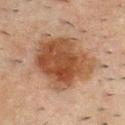Clinical impression:
Imaged during a routine full-body skin examination; the lesion was not biopsied and no histopathology is available.
Background:
The lesion is on the chest. A region of skin cropped from a whole-body photographic capture, roughly 15 mm wide. The patient is a male aged 48 to 52. The recorded lesion diameter is about 7.5 mm.